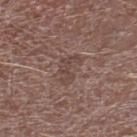Imaged during a routine full-body skin examination; the lesion was not biopsied and no histopathology is available.
A lesion tile, about 15 mm wide, cut from a 3D total-body photograph.
Located on the right lower leg.
A male subject roughly 75 years of age.